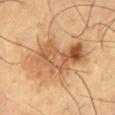Captured during whole-body skin photography for melanoma surveillance; the lesion was not biopsied. A lesion tile, about 15 mm wide, cut from a 3D total-body photograph. From the left thigh. The tile uses cross-polarized illumination. The total-body-photography lesion software estimated an area of roughly 24 mm², an eccentricity of roughly 0.85, and a shape-asymmetry score of about 0.35 (0 = symmetric). The analysis additionally found a mean CIELAB color near L≈58 a*≈22 b*≈38, a lesion–skin lightness drop of about 13, and a lesion-to-skin contrast of about 8 (normalized; higher = more distinct). The analysis additionally found a classifier nevus-likeness of about 10/100 and a detector confidence of about 100 out of 100 that the crop contains a lesion. A male patient roughly 65 years of age.